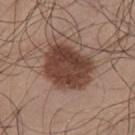Case summary:
– workup: no biopsy performed (imaged during a skin exam)
– image source: 15 mm crop, total-body photography
– location: the chest
– subject: male, about 25 years old